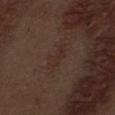biopsy status: catalogued during a skin exam; not biopsied | image source: ~15 mm tile from a whole-body skin photo | subject: male, in their 70s | lesion size: ≈4 mm | location: the abdomen | automated metrics: a within-lesion color-variation index near 1/10; a nevus-likeness score of about 5/100 and a lesion-detection confidence of about 100/100 | illumination: white-light.